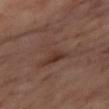{"biopsy_status": "not biopsied; imaged during a skin examination", "lighting": "cross-polarized", "automated_metrics": {"area_mm2_approx": 14.0, "eccentricity": 0.55, "shape_asymmetry": 0.7, "border_irregularity_0_10": 8.0, "color_variation_0_10": 5.5, "peripheral_color_asymmetry": 2.0, "nevus_likeness_0_100": 0, "lesion_detection_confidence_0_100": 100}, "patient": {"sex": "female", "age_approx": 55}, "site": "right thigh", "image": {"source": "total-body photography crop", "field_of_view_mm": 15}, "lesion_size": {"long_diameter_mm_approx": 5.5}}